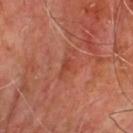Impression:
Imaged during a routine full-body skin examination; the lesion was not biopsied and no histopathology is available.
Clinical summary:
On the chest. Automated image analysis of the tile measured a lesion area of about 2.5 mm² and two-axis asymmetry of about 0.5. And it measured an automated nevus-likeness rating near 0 out of 100 and lesion-presence confidence of about 95/100. Imaged with cross-polarized lighting. About 2.5 mm across. Cropped from a whole-body photographic skin survey; the tile spans about 15 mm. The patient is a male approximately 65 years of age.